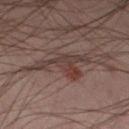Case summary:
• biopsy status — no biopsy performed (imaged during a skin exam)
• image — total-body-photography crop, ~15 mm field of view
• body site — the leg
• subject — male, aged 38–42